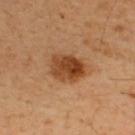Imaged during a routine full-body skin examination; the lesion was not biopsied and no histopathology is available.
Imaged with cross-polarized lighting.
Automated tile analysis of the lesion measured about 12 CIELAB-L* units darker than the surrounding skin. The software also gave a border-irregularity index near 2/10 and internal color variation of about 6.5 on a 0–10 scale.
A roughly 15 mm field-of-view crop from a total-body skin photograph.
The recorded lesion diameter is about 4 mm.
Located on the upper back.
A male subject approximately 50 years of age.